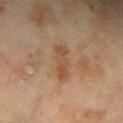- workup — imaged on a skin check; not biopsied
- imaging modality — 15 mm crop, total-body photography
- patient — female, about 60 years old
- illumination — cross-polarized illumination
- lesion diameter — ~3.5 mm (longest diameter)
- automated metrics — an area of roughly 6 mm² and a shape eccentricity near 0.9; an automated nevus-likeness rating near 0 out of 100 and a detector confidence of about 100 out of 100 that the crop contains a lesion
- anatomic site — the right forearm